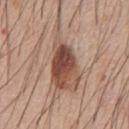A male subject about 60 years old.
An algorithmic analysis of the crop reported a border-irregularity index near 5/10, internal color variation of about 8.5 on a 0–10 scale, and a peripheral color-asymmetry measure near 2.5.
The lesion is located on the chest.
This is a white-light tile.
A 15 mm close-up extracted from a 3D total-body photography capture.